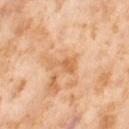Clinical impression:
This lesion was catalogued during total-body skin photography and was not selected for biopsy.
Acquisition and patient details:
The tile uses cross-polarized illumination. A female patient, aged around 55. On the right thigh. Longest diameter approximately 3.5 mm. The lesion-visualizer software estimated a shape eccentricity near 0.9 and a symmetry-axis asymmetry near 0.55. It also reported border irregularity of about 6 on a 0–10 scale and a peripheral color-asymmetry measure near 0.5. It also reported a nevus-likeness score of about 0/100 and a detector confidence of about 100 out of 100 that the crop contains a lesion. Cropped from a whole-body photographic skin survey; the tile spans about 15 mm.